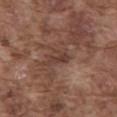Part of a total-body skin-imaging series; this lesion was reviewed on a skin check and was not flagged for biopsy.
On the abdomen.
A close-up tile cropped from a whole-body skin photograph, about 15 mm across.
A male subject in their mid-70s.
The lesion-visualizer software estimated internal color variation of about 1 on a 0–10 scale.
Imaged with white-light lighting.
Measured at roughly 3 mm in maximum diameter.